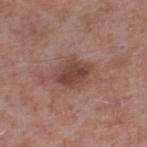Notes:
• biopsy status: total-body-photography surveillance lesion; no biopsy
• patient: male, aged approximately 55
• imaging modality: 15 mm crop, total-body photography
• tile lighting: white-light illumination
• location: the right lower leg